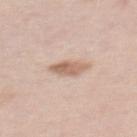Assessment: Imaged during a routine full-body skin examination; the lesion was not biopsied and no histopathology is available. Clinical summary: The lesion is located on the back. A female patient, about 35 years old. A roughly 15 mm field-of-view crop from a total-body skin photograph.